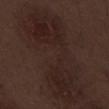Q: Was a biopsy performed?
A: total-body-photography surveillance lesion; no biopsy
Q: What lighting was used for the tile?
A: white-light illumination
Q: What are the patient's age and sex?
A: male, aged 68–72
Q: How large is the lesion?
A: ~13.5 mm (longest diameter)
Q: How was this image acquired?
A: ~15 mm crop, total-body skin-cancer survey
Q: Where on the body is the lesion?
A: the left thigh